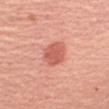{"biopsy_status": "not biopsied; imaged during a skin examination", "site": "right upper arm", "lesion_size": {"long_diameter_mm_approx": 3.5}, "lighting": "white-light", "image": {"source": "total-body photography crop", "field_of_view_mm": 15}, "patient": {"sex": "female", "age_approx": 55}, "automated_metrics": {"lesion_detection_confidence_0_100": 100}}